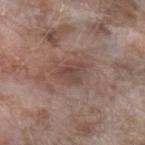Clinical impression:
The lesion was tiled from a total-body skin photograph and was not biopsied.
Clinical summary:
A region of skin cropped from a whole-body photographic capture, roughly 15 mm wide. On the left forearm. The patient is a female in their mid-70s. The recorded lesion diameter is about 3.5 mm. Captured under white-light illumination.